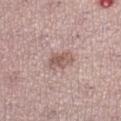Assessment:
Captured during whole-body skin photography for melanoma surveillance; the lesion was not biopsied.
Image and clinical context:
Cropped from a whole-body photographic skin survey; the tile spans about 15 mm. About 3 mm across. On the left lower leg. The patient is a female in their mid- to late 50s. The lesion-visualizer software estimated a lesion area of about 5.5 mm² and an outline eccentricity of about 0.7 (0 = round, 1 = elongated). The software also gave about 10 CIELAB-L* units darker than the surrounding skin and a normalized lesion–skin contrast near 7. The software also gave border irregularity of about 2.5 on a 0–10 scale, internal color variation of about 4 on a 0–10 scale, and radial color variation of about 1.5. The tile uses white-light illumination.